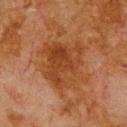The lesion was photographed on a routine skin check and not biopsied; there is no pathology result. A lesion tile, about 15 mm wide, cut from a 3D total-body photograph. The lesion's longest dimension is about 6 mm. Captured under cross-polarized illumination. The patient is a male aged approximately 80. Located on the upper back.